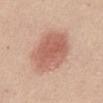| feature | finding |
|---|---|
| notes | imaged on a skin check; not biopsied |
| patient | female, roughly 50 years of age |
| location | the abdomen |
| acquisition | ~15 mm tile from a whole-body skin photo |